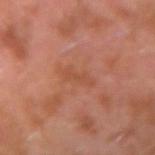| key | value |
|---|---|
| biopsy status | catalogued during a skin exam; not biopsied |
| patient | male, aged approximately 30 |
| location | the left arm |
| image source | total-body-photography crop, ~15 mm field of view |